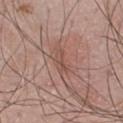notes — imaged on a skin check; not biopsied | image-analysis metrics — border irregularity of about 4.5 on a 0–10 scale, a within-lesion color-variation index near 0.5/10, and radial color variation of about 0 | tile lighting — white-light illumination | diameter — about 3 mm | body site — the upper back | subject — male, aged around 45 | imaging modality — ~15 mm tile from a whole-body skin photo.